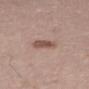notes: no biopsy performed (imaged during a skin exam) | location: the left thigh | lighting: white-light | TBP lesion metrics: a lesion area of about 5.5 mm² and a symmetry-axis asymmetry near 0.2; a border-irregularity rating of about 2/10, internal color variation of about 2.5 on a 0–10 scale, and peripheral color asymmetry of about 1; a lesion-detection confidence of about 100/100 | image: total-body-photography crop, ~15 mm field of view | lesion diameter: ~2.5 mm (longest diameter) | subject: male, in their mid-60s.